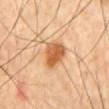Imaged during a routine full-body skin examination; the lesion was not biopsied and no histopathology is available.
The patient is a male about 60 years old.
Imaged with cross-polarized lighting.
A region of skin cropped from a whole-body photographic capture, roughly 15 mm wide.
Located on the mid back.
Measured at roughly 4 mm in maximum diameter.